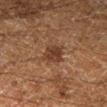Q: Was a biopsy performed?
A: no biopsy performed (imaged during a skin exam)
Q: How was this image acquired?
A: total-body-photography crop, ~15 mm field of view
Q: Illumination type?
A: cross-polarized illumination
Q: What did automated image analysis measure?
A: a shape eccentricity near 0.65 and two-axis asymmetry of about 0.2; a mean CIELAB color near L≈29 a*≈16 b*≈24, roughly 7 lightness units darker than nearby skin, and a lesion-to-skin contrast of about 8 (normalized; higher = more distinct); a border-irregularity rating of about 2/10, a color-variation rating of about 2/10, and peripheral color asymmetry of about 0.5
Q: What is the lesion's diameter?
A: ~3.5 mm (longest diameter)
Q: Patient demographics?
A: male, aged 58–62
Q: Where on the body is the lesion?
A: the right lower leg